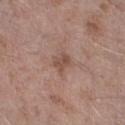{"biopsy_status": "not biopsied; imaged during a skin examination", "site": "right forearm", "image": {"source": "total-body photography crop", "field_of_view_mm": 15}, "patient": {"sex": "male", "age_approx": 70}, "lesion_size": {"long_diameter_mm_approx": 2.5}}